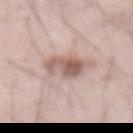Notes:
* body site — the abdomen
* subject — male, aged 68–72
* image source — total-body-photography crop, ~15 mm field of view